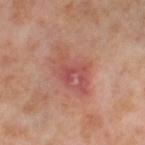Impression:
No biopsy was performed on this lesion — it was imaged during a full skin examination and was not determined to be concerning.
Context:
The recorded lesion diameter is about 5.5 mm. This is a cross-polarized tile. The lesion is on the leg. The subject is a female about 55 years old. A close-up tile cropped from a whole-body skin photograph, about 15 mm across.